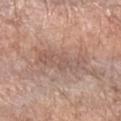| feature | finding |
|---|---|
| follow-up | no biopsy performed (imaged during a skin exam) |
| acquisition | ~15 mm crop, total-body skin-cancer survey |
| subject | female, aged 53 to 57 |
| automated metrics | an average lesion color of about L≈56 a*≈18 b*≈25 (CIELAB) and roughly 8 lightness units darker than nearby skin |
| body site | the left forearm |